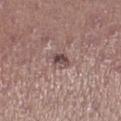Q: Lesion location?
A: the left lower leg
Q: What is the imaging modality?
A: ~15 mm crop, total-body skin-cancer survey
Q: What is the lesion's diameter?
A: about 2.5 mm
Q: What are the patient's age and sex?
A: female, roughly 20 years of age